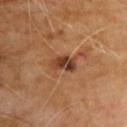| feature | finding |
|---|---|
| follow-up | catalogued during a skin exam; not biopsied |
| body site | the chest |
| patient | male, approximately 70 years of age |
| imaging modality | 15 mm crop, total-body photography |